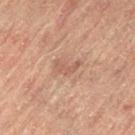Recorded during total-body skin imaging; not selected for excision or biopsy. Approximately 3 mm at its widest. A female patient aged 78–82. From the right leg. This is a cross-polarized tile. A 15 mm crop from a total-body photograph taken for skin-cancer surveillance. The total-body-photography lesion software estimated an area of roughly 3 mm² and a symmetry-axis asymmetry near 0.6. And it measured a lesion color around L≈51 a*≈20 b*≈26 in CIELAB and a lesion-to-skin contrast of about 5 (normalized; higher = more distinct). And it measured a within-lesion color-variation index near 0/10 and peripheral color asymmetry of about 0.